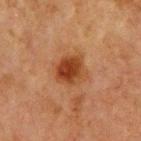This lesion was catalogued during total-body skin photography and was not selected for biopsy.
Captured under cross-polarized illumination.
A roughly 15 mm field-of-view crop from a total-body skin photograph.
On the chest.
The patient is a male about 65 years old.